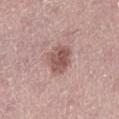| feature | finding |
|---|---|
| biopsy status | catalogued during a skin exam; not biopsied |
| patient | female, in their 40s |
| site | the left lower leg |
| size | ≈3.5 mm |
| imaging modality | ~15 mm crop, total-body skin-cancer survey |